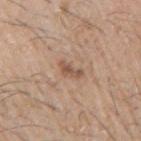biopsy status: total-body-photography surveillance lesion; no biopsy | anatomic site: the right upper arm | subject: male, approximately 65 years of age | diameter: ~3 mm (longest diameter) | acquisition: 15 mm crop, total-body photography.